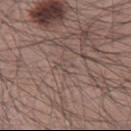Q: Was this lesion biopsied?
A: total-body-photography surveillance lesion; no biopsy